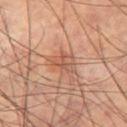Notes:
* lesion diameter — ≈2.5 mm
* subject — male, aged 58 to 62
* illumination — cross-polarized illumination
* acquisition — 15 mm crop, total-body photography
* TBP lesion metrics — a lesion area of about 3.5 mm² and two-axis asymmetry of about 0.6; an average lesion color of about L≈53 a*≈24 b*≈31 (CIELAB) and a lesion–skin lightness drop of about 8; a border-irregularity rating of about 5.5/10, a color-variation rating of about 0/10, and a peripheral color-asymmetry measure near 0
* site — the right thigh